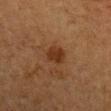Q: Was this lesion biopsied?
A: imaged on a skin check; not biopsied
Q: What did automated image analysis measure?
A: a lesion area of about 5 mm² and a symmetry-axis asymmetry near 0.3; an average lesion color of about L≈29 a*≈20 b*≈29 (CIELAB), about 8 CIELAB-L* units darker than the surrounding skin, and a normalized border contrast of about 8; a classifier nevus-likeness of about 85/100 and lesion-presence confidence of about 100/100
Q: How was the tile lit?
A: cross-polarized
Q: What are the patient's age and sex?
A: female, aged 38–42
Q: What is the lesion's diameter?
A: ≈3 mm
Q: What is the anatomic site?
A: the right forearm
Q: How was this image acquired?
A: 15 mm crop, total-body photography